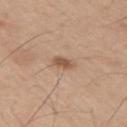Assessment: Captured during whole-body skin photography for melanoma surveillance; the lesion was not biopsied. Acquisition and patient details: Approximately 2.5 mm at its widest. The subject is a male roughly 55 years of age. From the right upper arm. A roughly 15 mm field-of-view crop from a total-body skin photograph.